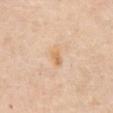site: abdomen
image:
  source: total-body photography crop
  field_of_view_mm: 15
patient:
  sex: female
  age_approx: 65